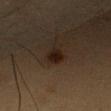Acquisition and patient details:
The lesion is located on the right upper arm. The tile uses cross-polarized illumination. Measured at roughly 2.5 mm in maximum diameter. The subject is a male in their mid-50s. Automated image analysis of the tile measured an average lesion color of about L≈16 a*≈13 b*≈18 (CIELAB) and a lesion–skin lightness drop of about 8. The analysis additionally found a classifier nevus-likeness of about 95/100 and a detector confidence of about 100 out of 100 that the crop contains a lesion. Cropped from a total-body skin-imaging series; the visible field is about 15 mm.